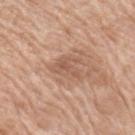Part of a total-body skin-imaging series; this lesion was reviewed on a skin check and was not flagged for biopsy. A region of skin cropped from a whole-body photographic capture, roughly 15 mm wide. The subject is a female in their mid-70s. On the right upper arm. Captured under white-light illumination. The lesion's longest dimension is about 3 mm.